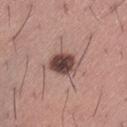* lesion diameter — ≈3.5 mm
* illumination — white-light
* anatomic site — the left thigh
* patient — male, in their mid- to late 40s
* TBP lesion metrics — a footprint of about 8 mm² and an eccentricity of roughly 0.65; a lesion color around L≈44 a*≈19 b*≈21 in CIELAB, roughly 17 lightness units darker than nearby skin, and a lesion-to-skin contrast of about 12.5 (normalized; higher = more distinct); a border-irregularity rating of about 1/10 and a peripheral color-asymmetry measure near 1.5; lesion-presence confidence of about 100/100
* imaging modality — ~15 mm tile from a whole-body skin photo